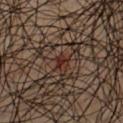| field | value |
|---|---|
| workup | catalogued during a skin exam; not biopsied |
| location | the front of the torso |
| lesion size | about 2 mm |
| lighting | cross-polarized illumination |
| image source | ~15 mm crop, total-body skin-cancer survey |
| patient | male, aged approximately 50 |